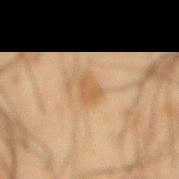<tbp_lesion>
  <biopsy_status>not biopsied; imaged during a skin examination</biopsy_status>
  <automated_metrics>
    <nevus_likeness_0_100>5</nevus_likeness_0_100>
    <lesion_detection_confidence_0_100>100</lesion_detection_confidence_0_100>
  </automated_metrics>
  <lighting>cross-polarized</lighting>
  <lesion_size>
    <long_diameter_mm_approx>3.0</long_diameter_mm_approx>
  </lesion_size>
  <site>mid back</site>
  <image>
    <source>total-body photography crop</source>
    <field_of_view_mm>15</field_of_view_mm>
  </image>
  <patient>
    <sex>male</sex>
    <age_approx>50</age_approx>
  </patient>
</tbp_lesion>